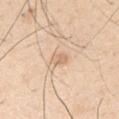Q: Was this lesion biopsied?
A: total-body-photography surveillance lesion; no biopsy
Q: Automated lesion metrics?
A: about 8 CIELAB-L* units darker than the surrounding skin and a normalized lesion–skin contrast near 5.5; a border-irregularity rating of about 2/10, a color-variation rating of about 2/10, and a peripheral color-asymmetry measure near 1; a classifier nevus-likeness of about 0/100 and a detector confidence of about 100 out of 100 that the crop contains a lesion
Q: What are the patient's age and sex?
A: male, in their 30s
Q: Lesion location?
A: the left upper arm
Q: How large is the lesion?
A: ~2.5 mm (longest diameter)
Q: What is the imaging modality?
A: 15 mm crop, total-body photography
Q: What lighting was used for the tile?
A: white-light